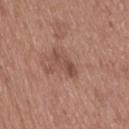No biopsy was performed on this lesion — it was imaged during a full skin examination and was not determined to be concerning. Captured under white-light illumination. A close-up tile cropped from a whole-body skin photograph, about 15 mm across. The total-body-photography lesion software estimated a footprint of about 5.5 mm², a shape eccentricity near 0.9, and a shape-asymmetry score of about 0.25 (0 = symmetric). Measured at roughly 3.5 mm in maximum diameter. A male patient, roughly 40 years of age. The lesion is located on the upper back.